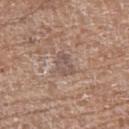<case>
  <biopsy_status>not biopsied; imaged during a skin examination</biopsy_status>
  <image>
    <source>total-body photography crop</source>
    <field_of_view_mm>15</field_of_view_mm>
  </image>
  <patient>
    <sex>female</sex>
    <age_approx>75</age_approx>
  </patient>
  <lighting>white-light</lighting>
  <site>left thigh</site>
  <lesion_size>
    <long_diameter_mm_approx>3.0</long_diameter_mm_approx>
  </lesion_size>
</case>